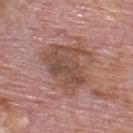Notes:
– notes · no biopsy performed (imaged during a skin exam)
– image · ~15 mm tile from a whole-body skin photo
– patient · male, aged approximately 75
– image-analysis metrics · a lesion area of about 21 mm², an outline eccentricity of about 0.65 (0 = round, 1 = elongated), and a shape-asymmetry score of about 0.35 (0 = symmetric); roughly 9 lightness units darker than nearby skin and a lesion-to-skin contrast of about 7 (normalized; higher = more distinct); a nevus-likeness score of about 0/100 and a lesion-detection confidence of about 100/100
– site · the upper back
– lesion size · about 6.5 mm
– tile lighting · white-light